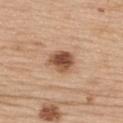A lesion tile, about 15 mm wide, cut from a 3D total-body photograph. A female patient, about 65 years old. The lesion is on the upper back.